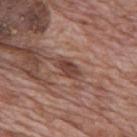Findings:
- workup · imaged on a skin check; not biopsied
- anatomic site · the mid back
- image-analysis metrics · border irregularity of about 2.5 on a 0–10 scale, a within-lesion color-variation index near 2/10, and radial color variation of about 0.5; a classifier nevus-likeness of about 15/100 and lesion-presence confidence of about 100/100
- image source · 15 mm crop, total-body photography
- diameter · about 3 mm
- subject · male, about 70 years old
- illumination · white-light